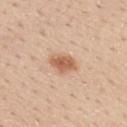No biopsy was performed on this lesion — it was imaged during a full skin examination and was not determined to be concerning. The tile uses white-light illumination. The lesion-visualizer software estimated a mean CIELAB color near L≈61 a*≈22 b*≈33, about 12 CIELAB-L* units darker than the surrounding skin, and a lesion-to-skin contrast of about 8 (normalized; higher = more distinct). The analysis additionally found an automated nevus-likeness rating near 95 out of 100 and a lesion-detection confidence of about 100/100. A male subject roughly 30 years of age. A lesion tile, about 15 mm wide, cut from a 3D total-body photograph. From the mid back.